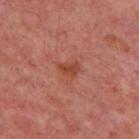Impression:
The lesion was photographed on a routine skin check and not biopsied; there is no pathology result.
Image and clinical context:
Automated tile analysis of the lesion measured an area of roughly 3.5 mm² and two-axis asymmetry of about 0.35. The software also gave a normalized lesion–skin contrast near 7. And it measured a classifier nevus-likeness of about 5/100 and lesion-presence confidence of about 100/100. Imaged with cross-polarized lighting. A 15 mm crop from a total-body photograph taken for skin-cancer surveillance. The lesion's longest dimension is about 2.5 mm. A subject about 55 years old. On the upper back.